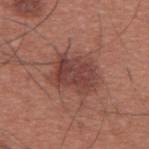Clinical impression: This lesion was catalogued during total-body skin photography and was not selected for biopsy. Acquisition and patient details: Approximately 5.5 mm at its widest. A male subject, aged 33–37. This image is a 15 mm lesion crop taken from a total-body photograph. The lesion is on the back. Automated image analysis of the tile measured an average lesion color of about L≈42 a*≈23 b*≈24 (CIELAB), a lesion–skin lightness drop of about 10, and a normalized lesion–skin contrast near 8. Captured under white-light illumination.